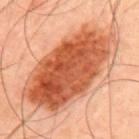Clinical impression:
Recorded during total-body skin imaging; not selected for excision or biopsy.
Clinical summary:
Located on the upper back. A male subject, in their 50s. Cropped from a whole-body photographic skin survey; the tile spans about 15 mm.